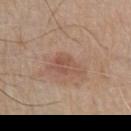The lesion was tiled from a total-body skin photograph and was not biopsied.
Imaged with white-light lighting.
Approximately 4 mm at its widest.
The total-body-photography lesion software estimated border irregularity of about 4 on a 0–10 scale, internal color variation of about 3.5 on a 0–10 scale, and a peripheral color-asymmetry measure near 1. The software also gave an automated nevus-likeness rating near 5 out of 100 and a lesion-detection confidence of about 100/100.
The subject is a male roughly 80 years of age.
The lesion is located on the chest.
This image is a 15 mm lesion crop taken from a total-body photograph.